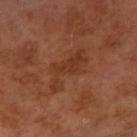Acquisition and patient details:
The recorded lesion diameter is about 6 mm. A male subject aged 53–57. A 15 mm close-up extracted from a 3D total-body photography capture. The lesion is on the right upper arm.